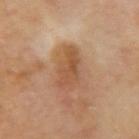* workup — total-body-photography surveillance lesion; no biopsy
* patient — male, aged around 70
* body site — the right upper arm
* lesion diameter — about 5.5 mm
* illumination — cross-polarized
* image source — ~15 mm crop, total-body skin-cancer survey